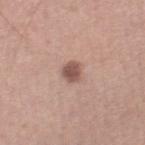- notes: imaged on a skin check; not biopsied
- imaging modality: ~15 mm tile from a whole-body skin photo
- lesion diameter: about 2.5 mm
- location: the right thigh
- illumination: white-light
- subject: male, aged around 50
- automated metrics: a footprint of about 4.5 mm², a shape eccentricity near 0.6, and a symmetry-axis asymmetry near 0.2; a classifier nevus-likeness of about 80/100 and lesion-presence confidence of about 100/100